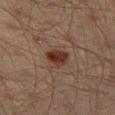The lesion was photographed on a routine skin check and not biopsied; there is no pathology result.
On the left thigh.
A male subject in their mid- to late 40s.
A 15 mm close-up tile from a total-body photography series done for melanoma screening.